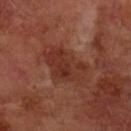Part of a total-body skin-imaging series; this lesion was reviewed on a skin check and was not flagged for biopsy.
The patient is a male in their 70s.
The total-body-photography lesion software estimated a classifier nevus-likeness of about 0/100 and lesion-presence confidence of about 100/100.
Captured under cross-polarized illumination.
A 15 mm close-up tile from a total-body photography series done for melanoma screening.
The lesion's longest dimension is about 5.5 mm.
Located on the right forearm.